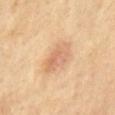Q: Was this lesion biopsied?
A: total-body-photography surveillance lesion; no biopsy
Q: Patient demographics?
A: male, aged approximately 60
Q: What is the imaging modality?
A: total-body-photography crop, ~15 mm field of view
Q: Where on the body is the lesion?
A: the right upper arm